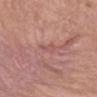Captured during whole-body skin photography for melanoma surveillance; the lesion was not biopsied. A female patient, roughly 70 years of age. This image is a 15 mm lesion crop taken from a total-body photograph. The lesion is located on the left forearm.